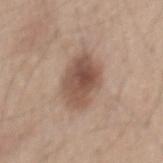biopsy_status: not biopsied; imaged during a skin examination
image:
  source: total-body photography crop
  field_of_view_mm: 15
site: mid back
lighting: white-light
lesion_size:
  long_diameter_mm_approx: 6.0
patient:
  sex: male
  age_approx: 45